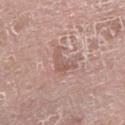Q: Was a biopsy performed?
A: catalogued during a skin exam; not biopsied
Q: What are the patient's age and sex?
A: male, in their mid- to late 70s
Q: How was this image acquired?
A: total-body-photography crop, ~15 mm field of view
Q: What is the anatomic site?
A: the left lower leg